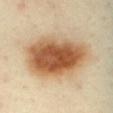No biopsy was performed on this lesion — it was imaged during a full skin examination and was not determined to be concerning. Located on the mid back. A close-up tile cropped from a whole-body skin photograph, about 15 mm across. A female subject in their 40s. About 9 mm across. Imaged with cross-polarized lighting.